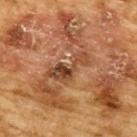biopsy status — catalogued during a skin exam; not biopsied | diameter — about 5.5 mm | anatomic site — the upper back | lighting — cross-polarized illumination | patient — male, aged 83 or over | acquisition — ~15 mm tile from a whole-body skin photo | image-analysis metrics — a lesion area of about 9 mm², an eccentricity of roughly 0.9, and a shape-asymmetry score of about 0.55 (0 = symmetric); a mean CIELAB color near L≈39 a*≈20 b*≈30, about 9 CIELAB-L* units darker than the surrounding skin, and a normalized lesion–skin contrast near 7.5.